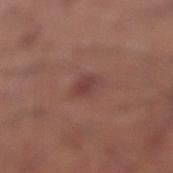Assessment:
This lesion was catalogued during total-body skin photography and was not selected for biopsy.
Image and clinical context:
Automated image analysis of the tile measured a shape eccentricity near 0.6 and a shape-asymmetry score of about 0.25 (0 = symmetric). And it measured an average lesion color of about L≈42 a*≈22 b*≈23 (CIELAB), about 7 CIELAB-L* units darker than the surrounding skin, and a normalized border contrast of about 6. It also reported border irregularity of about 2 on a 0–10 scale, a color-variation rating of about 2.5/10, and radial color variation of about 1. The software also gave a classifier nevus-likeness of about 55/100. The patient is a male aged approximately 45. A 15 mm crop from a total-body photograph taken for skin-cancer surveillance. Approximately 3 mm at its widest. Located on the leg. Imaged with white-light lighting.